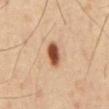<lesion>
  <biopsy_status>not biopsied; imaged during a skin examination</biopsy_status>
  <lighting>cross-polarized</lighting>
  <lesion_size>
    <long_diameter_mm_approx>3.5</long_diameter_mm_approx>
  </lesion_size>
  <automated_metrics>
    <eccentricity>0.85</eccentricity>
    <shape_asymmetry>0.15</shape_asymmetry>
    <cielab_L>51</cielab_L>
    <cielab_a>23</cielab_a>
    <cielab_b>33</cielab_b>
    <vs_skin_contrast_norm>12.0</vs_skin_contrast_norm>
    <border_irregularity_0_10>1.5</border_irregularity_0_10>
  </automated_metrics>
  <image>
    <source>total-body photography crop</source>
    <field_of_view_mm>15</field_of_view_mm>
  </image>
  <site>front of the torso</site>
  <patient>
    <sex>male</sex>
    <age_approx>55</age_approx>
  </patient>
</lesion>